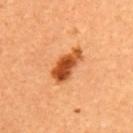The lesion was photographed on a routine skin check and not biopsied; there is no pathology result.